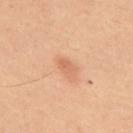Q: Was this lesion biopsied?
A: total-body-photography surveillance lesion; no biopsy